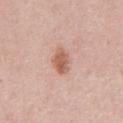{
  "biopsy_status": "not biopsied; imaged during a skin examination",
  "lighting": "white-light",
  "site": "chest",
  "patient": {
    "sex": "male",
    "age_approx": 55
  },
  "lesion_size": {
    "long_diameter_mm_approx": 3.5
  },
  "image": {
    "source": "total-body photography crop",
    "field_of_view_mm": 15
  },
  "automated_metrics": {
    "area_mm2_approx": 6.0,
    "eccentricity": 0.8,
    "shape_asymmetry": 0.15,
    "peripheral_color_asymmetry": 1.0,
    "nevus_likeness_0_100": 90,
    "lesion_detection_confidence_0_100": 100
  }
}